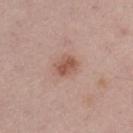follow-up = catalogued during a skin exam; not biopsied | body site = the leg | patient = male, in their mid- to late 50s | acquisition = total-body-photography crop, ~15 mm field of view | lighting = white-light | lesion size = ≈3 mm.